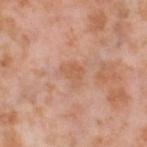Assessment: Part of a total-body skin-imaging series; this lesion was reviewed on a skin check and was not flagged for biopsy. Context: A female subject in their mid-50s. A close-up tile cropped from a whole-body skin photograph, about 15 mm across. On the left thigh.